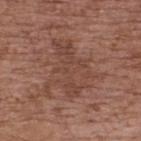Clinical summary: The patient is a female approximately 65 years of age. The lesion's longest dimension is about 6.5 mm. Captured under white-light illumination. Cropped from a total-body skin-imaging series; the visible field is about 15 mm. On the upper back.